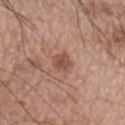• follow-up · imaged on a skin check; not biopsied
• image source · 15 mm crop, total-body photography
• location · the left upper arm
• subject · male, aged 63–67